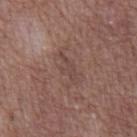follow-up = catalogued during a skin exam; not biopsied
site = the abdomen
diameter = ~4 mm (longest diameter)
illumination = white-light
patient = male, aged approximately 70
acquisition = total-body-photography crop, ~15 mm field of view